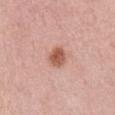Impression:
No biopsy was performed on this lesion — it was imaged during a full skin examination and was not determined to be concerning.
Background:
Imaged with white-light lighting. Automated image analysis of the tile measured a lesion area of about 5.5 mm², an eccentricity of roughly 0.5, and a symmetry-axis asymmetry near 0.25. The analysis additionally found a lesion color around L≈57 a*≈25 b*≈30 in CIELAB, roughly 13 lightness units darker than nearby skin, and a normalized border contrast of about 9. It also reported a border-irregularity index near 2/10 and a color-variation rating of about 3/10. The software also gave a detector confidence of about 100 out of 100 that the crop contains a lesion. About 3 mm across. Located on the abdomen. The patient is a female aged 58–62. A region of skin cropped from a whole-body photographic capture, roughly 15 mm wide.